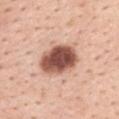Clinical impression:
The lesion was tiled from a total-body skin photograph and was not biopsied.
Background:
The total-body-photography lesion software estimated an average lesion color of about L≈52 a*≈24 b*≈27 (CIELAB) and about 22 CIELAB-L* units darker than the surrounding skin. The analysis additionally found a border-irregularity index near 1.5/10, internal color variation of about 5.5 on a 0–10 scale, and a peripheral color-asymmetry measure near 1.5. The software also gave an automated nevus-likeness rating near 90 out of 100 and lesion-presence confidence of about 100/100. About 5 mm across. The lesion is on the upper back. Cropped from a total-body skin-imaging series; the visible field is about 15 mm. The patient is a female aged around 45.